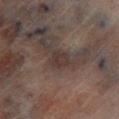workup: total-body-photography surveillance lesion; no biopsy
site: the left lower leg
subject: male, in their mid-60s
image: 15 mm crop, total-body photography
lesion size: ≈2.5 mm